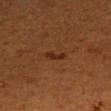The lesion was tiled from a total-body skin photograph and was not biopsied. From the left forearm. A female subject, aged around 55. A region of skin cropped from a whole-body photographic capture, roughly 15 mm wide. The tile uses cross-polarized illumination.